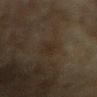Findings:
– notes · no biopsy performed (imaged during a skin exam)
– illumination · cross-polarized illumination
– automated metrics · a mean CIELAB color near L≈22 a*≈9 b*≈19, a lesion–skin lightness drop of about 4, and a lesion-to-skin contrast of about 5.5 (normalized; higher = more distinct); a border-irregularity index near 4/10, a within-lesion color-variation index near 1/10, and radial color variation of about 0.5; a nevus-likeness score of about 0/100 and a lesion-detection confidence of about 80/100
– location · the right forearm
– patient · female, in their 70s
– acquisition · total-body-photography crop, ~15 mm field of view
– diameter · ~3.5 mm (longest diameter)